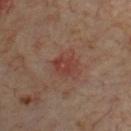Measured at roughly 3 mm in maximum diameter. A male subject in their 70s. From the chest. Cropped from a total-body skin-imaging series; the visible field is about 15 mm. Automated tile analysis of the lesion measured an area of roughly 5.5 mm², an outline eccentricity of about 0.65 (0 = round, 1 = elongated), and a shape-asymmetry score of about 0.2 (0 = symmetric). The analysis additionally found a lesion–skin lightness drop of about 6 and a lesion-to-skin contrast of about 6 (normalized; higher = more distinct). The tile uses cross-polarized illumination.